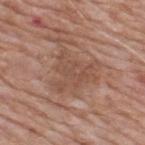Assessment:
This lesion was catalogued during total-body skin photography and was not selected for biopsy.
Image and clinical context:
Located on the upper back. A male patient aged 58 to 62. Cropped from a whole-body photographic skin survey; the tile spans about 15 mm. The recorded lesion diameter is about 4.5 mm. The total-body-photography lesion software estimated an average lesion color of about L≈50 a*≈20 b*≈28 (CIELAB) and a normalized lesion–skin contrast near 5. It also reported a border-irregularity rating of about 8/10, a color-variation rating of about 2/10, and peripheral color asymmetry of about 0.5. The tile uses white-light illumination.